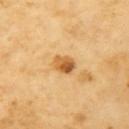Assessment: Recorded during total-body skin imaging; not selected for excision or biopsy. Image and clinical context: The lesion is located on the arm. The tile uses cross-polarized illumination. The patient is a female in their 70s. Automated tile analysis of the lesion measured a lesion area of about 5 mm², an outline eccentricity of about 0.65 (0 = round, 1 = elongated), and a symmetry-axis asymmetry near 0.25. And it measured an average lesion color of about L≈61 a*≈23 b*≈47 (CIELAB), a lesion–skin lightness drop of about 15, and a normalized border contrast of about 9. It also reported a nevus-likeness score of about 90/100. A 15 mm close-up extracted from a 3D total-body photography capture.